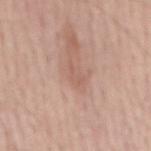Case summary:
• notes · total-body-photography surveillance lesion; no biopsy
• site · the back
• lighting · white-light illumination
• lesion size · ≈3 mm
• subject · male, about 55 years old
• acquisition · total-body-photography crop, ~15 mm field of view